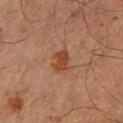{"biopsy_status": "not biopsied; imaged during a skin examination", "image": {"source": "total-body photography crop", "field_of_view_mm": 15}, "patient": {"sex": "male", "age_approx": 65}, "lesion_size": {"long_diameter_mm_approx": 3.0}, "site": "right lower leg"}